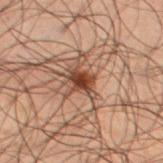Assessment: Imaged during a routine full-body skin examination; the lesion was not biopsied and no histopathology is available.